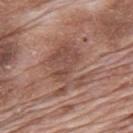Assessment: The lesion was photographed on a routine skin check and not biopsied; there is no pathology result. Acquisition and patient details: The recorded lesion diameter is about 6 mm. A 15 mm crop from a total-body photograph taken for skin-cancer surveillance. The patient is a male roughly 70 years of age. Imaged with white-light lighting. The lesion is on the mid back.